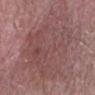Notes:
– notes: total-body-photography surveillance lesion; no biopsy
– location: the arm
– patient: male, about 60 years old
– image-analysis metrics: a lesion area of about 65 mm², an eccentricity of roughly 0.65, and a shape-asymmetry score of about 0.3 (0 = symmetric); a lesion color around L≈47 a*≈21 b*≈19 in CIELAB, roughly 6 lightness units darker than nearby skin, and a lesion-to-skin contrast of about 5 (normalized; higher = more distinct); a border-irregularity rating of about 5.5/10, internal color variation of about 4 on a 0–10 scale, and a peripheral color-asymmetry measure near 1.5
– image source: ~15 mm tile from a whole-body skin photo
– lesion size: ~12 mm (longest diameter)
– lighting: white-light